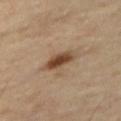notes: total-body-photography surveillance lesion; no biopsy | image source: total-body-photography crop, ~15 mm field of view | diameter: ≈4 mm | anatomic site: the right thigh | patient: female, in their 50s.